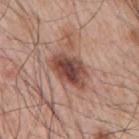biopsy_status: not biopsied; imaged during a skin examination